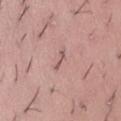Impression: No biopsy was performed on this lesion — it was imaged during a full skin examination and was not determined to be concerning. Acquisition and patient details: From the abdomen. This image is a 15 mm lesion crop taken from a total-body photograph. Imaged with white-light lighting. An algorithmic analysis of the crop reported a color-variation rating of about 0/10 and a peripheral color-asymmetry measure near 0. Measured at roughly 2.5 mm in maximum diameter. A female patient aged around 40.